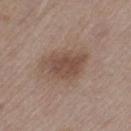Findings:
* notes — imaged on a skin check; not biopsied
* patient — female, aged approximately 40
* body site — the leg
* acquisition — 15 mm crop, total-body photography